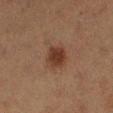| key | value |
|---|---|
| biopsy status | total-body-photography surveillance lesion; no biopsy |